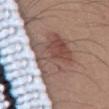No biopsy was performed on this lesion — it was imaged during a full skin examination and was not determined to be concerning.
The lesion is on the abdomen.
A 15 mm close-up tile from a total-body photography series done for melanoma screening.
Imaged with white-light lighting.
The subject is a male in their mid- to late 30s.
The lesion's longest dimension is about 6 mm.